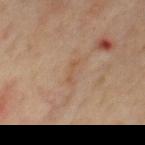A male patient, aged 58 to 62. The lesion's longest dimension is about 3 mm. This image is a 15 mm lesion crop taken from a total-body photograph. The lesion-visualizer software estimated a lesion area of about 2 mm², a shape eccentricity near 0.95, and two-axis asymmetry of about 0.5. It also reported a mean CIELAB color near L≈51 a*≈18 b*≈31 and a lesion-to-skin contrast of about 5.5 (normalized; higher = more distinct). The analysis additionally found border irregularity of about 7.5 on a 0–10 scale, a within-lesion color-variation index near 0/10, and a peripheral color-asymmetry measure near 0. On the chest.